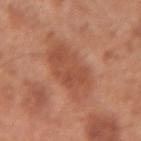<record>
  <biopsy_status>not biopsied; imaged during a skin examination</biopsy_status>
</record>